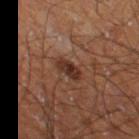follow-up = no biopsy performed (imaged during a skin exam) | body site = the leg | image = ~15 mm crop, total-body skin-cancer survey | subject = male, aged around 60.